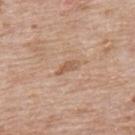Notes:
• workup: catalogued during a skin exam; not biopsied
• imaging modality: total-body-photography crop, ~15 mm field of view
• patient: male, aged around 65
• body site: the back
• TBP lesion metrics: an eccentricity of roughly 0.9 and two-axis asymmetry of about 0.4; a color-variation rating of about 0.5/10 and peripheral color asymmetry of about 0; a nevus-likeness score of about 0/100 and a lesion-detection confidence of about 100/100
• lesion size: ≈3 mm
• illumination: white-light